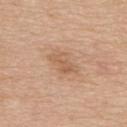Clinical impression:
The lesion was photographed on a routine skin check and not biopsied; there is no pathology result.
Background:
A male patient, in their mid-70s. The lesion is on the upper back. The lesion-visualizer software estimated border irregularity of about 3.5 on a 0–10 scale, a within-lesion color-variation index near 3.5/10, and peripheral color asymmetry of about 1. The software also gave an automated nevus-likeness rating near 5 out of 100 and a lesion-detection confidence of about 100/100. A roughly 15 mm field-of-view crop from a total-body skin photograph.